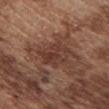biopsy_status: not biopsied; imaged during a skin examination
lighting: white-light
patient:
  sex: male
  age_approx: 75
lesion_size:
  long_diameter_mm_approx: 4.5
image:
  source: total-body photography crop
  field_of_view_mm: 15
site: chest